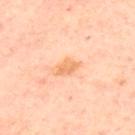Q: Is there a histopathology result?
A: catalogued during a skin exam; not biopsied
Q: What is the imaging modality?
A: ~15 mm crop, total-body skin-cancer survey
Q: Lesion location?
A: the upper back
Q: What lighting was used for the tile?
A: cross-polarized illumination
Q: Lesion size?
A: about 3 mm
Q: Patient demographics?
A: in their mid- to late 50s
Q: Automated lesion metrics?
A: an area of roughly 4 mm², a shape eccentricity near 0.8, and two-axis asymmetry of about 0.3; an average lesion color of about L≈73 a*≈25 b*≈42 (CIELAB), about 9 CIELAB-L* units darker than the surrounding skin, and a lesion-to-skin contrast of about 7 (normalized; higher = more distinct); an automated nevus-likeness rating near 0 out of 100 and lesion-presence confidence of about 100/100